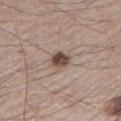Imaged during a routine full-body skin examination; the lesion was not biopsied and no histopathology is available. The tile uses white-light illumination. Cropped from a total-body skin-imaging series; the visible field is about 15 mm. A male patient aged 73 to 77. The total-body-photography lesion software estimated an area of roughly 4.5 mm², a shape eccentricity near 0.55, and a symmetry-axis asymmetry near 0.2. It also reported a lesion color around L≈46 a*≈15 b*≈20 in CIELAB, about 16 CIELAB-L* units darker than the surrounding skin, and a normalized lesion–skin contrast near 11.5. And it measured an automated nevus-likeness rating near 95 out of 100 and lesion-presence confidence of about 100/100. From the left lower leg. Longest diameter approximately 2.5 mm.